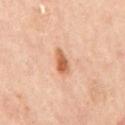<case>
<biopsy_status>not biopsied; imaged during a skin examination</biopsy_status>
<lighting>cross-polarized</lighting>
<site>mid back</site>
<image>
  <source>total-body photography crop</source>
  <field_of_view_mm>15</field_of_view_mm>
</image>
<lesion_size>
  <long_diameter_mm_approx>3.0</long_diameter_mm_approx>
</lesion_size>
<patient>
  <sex>female</sex>
  <age_approx>60</age_approx>
</patient>
</case>